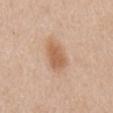Recorded during total-body skin imaging; not selected for excision or biopsy. The lesion is located on the mid back. The subject is a male aged 58–62. A lesion tile, about 15 mm wide, cut from a 3D total-body photograph.